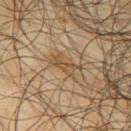The lesion was photographed on a routine skin check and not biopsied; there is no pathology result.
A male subject, aged 63 to 67.
A close-up tile cropped from a whole-body skin photograph, about 15 mm across.
The lesion is on the upper back.
About 4 mm across.
Imaged with cross-polarized lighting.